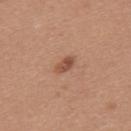Assessment:
Part of a total-body skin-imaging series; this lesion was reviewed on a skin check and was not flagged for biopsy.
Clinical summary:
An algorithmic analysis of the crop reported a symmetry-axis asymmetry near 0.25. A female patient, roughly 35 years of age. The lesion's longest dimension is about 2.5 mm. A 15 mm crop from a total-body photograph taken for skin-cancer surveillance. On the upper back.